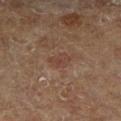The lesion was photographed on a routine skin check and not biopsied; there is no pathology result.